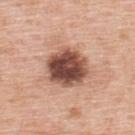Case summary:
– follow-up — no biopsy performed (imaged during a skin exam)
– automated metrics — a border-irregularity rating of about 1.5/10, internal color variation of about 8 on a 0–10 scale, and a peripheral color-asymmetry measure near 2.5; lesion-presence confidence of about 100/100
– lighting — white-light illumination
– image source — total-body-photography crop, ~15 mm field of view
– lesion diameter — ~5 mm (longest diameter)
– patient — male, aged 58–62
– body site — the upper back